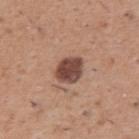Imaged during a routine full-body skin examination; the lesion was not biopsied and no histopathology is available.
Cropped from a total-body skin-imaging series; the visible field is about 15 mm.
On the arm.
The patient is a male about 50 years old.
The lesion's longest dimension is about 3.5 mm.